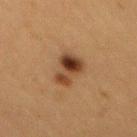notes=imaged on a skin check; not biopsied
image=~15 mm crop, total-body skin-cancer survey
tile lighting=cross-polarized
automated metrics=a lesion color around L≈34 a*≈18 b*≈29 in CIELAB and a lesion–skin lightness drop of about 12; an automated nevus-likeness rating near 100 out of 100 and lesion-presence confidence of about 100/100
subject=female, aged 38–42
body site=the mid back